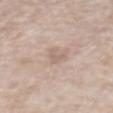The total-body-photography lesion software estimated a lesion area of about 3 mm², an outline eccentricity of about 0.85 (0 = round, 1 = elongated), and a symmetry-axis asymmetry near 0.55. It also reported a lesion–skin lightness drop of about 7 and a normalized lesion–skin contrast near 4.5. The analysis additionally found a color-variation rating of about 0/10 and peripheral color asymmetry of about 0. The analysis additionally found a lesion-detection confidence of about 90/100. A region of skin cropped from a whole-body photographic capture, roughly 15 mm wide. Longest diameter approximately 3 mm. A male subject, in their 70s. Imaged with white-light lighting. From the front of the torso.